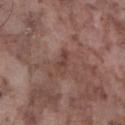Assessment:
The lesion was tiled from a total-body skin photograph and was not biopsied.
Clinical summary:
The subject is a male in their mid-70s. Longest diameter approximately 3 mm. The lesion is located on the left lower leg. This is a white-light tile. An algorithmic analysis of the crop reported a border-irregularity rating of about 4/10, a color-variation rating of about 1/10, and radial color variation of about 0. A 15 mm crop from a total-body photograph taken for skin-cancer surveillance.